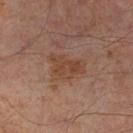No biopsy was performed on this lesion — it was imaged during a full skin examination and was not determined to be concerning.
Cropped from a whole-body photographic skin survey; the tile spans about 15 mm.
Approximately 4.5 mm at its widest.
A male patient, approximately 65 years of age.
The lesion is located on the left thigh.
Automated tile analysis of the lesion measured a mean CIELAB color near L≈42 a*≈19 b*≈28, roughly 6 lightness units darker than nearby skin, and a normalized lesion–skin contrast near 6.5. The software also gave a border-irregularity rating of about 4.5/10 and radial color variation of about 1. It also reported a nevus-likeness score of about 10/100 and a detector confidence of about 100 out of 100 that the crop contains a lesion.